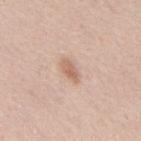Impression:
No biopsy was performed on this lesion — it was imaged during a full skin examination and was not determined to be concerning.
Clinical summary:
A 15 mm close-up extracted from a 3D total-body photography capture. The patient is a male in their mid- to late 40s. On the mid back. Imaged with white-light lighting. Measured at roughly 3 mm in maximum diameter.